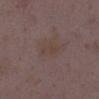biopsy status: catalogued during a skin exam; not biopsied | automated lesion analysis: a mean CIELAB color near L≈40 a*≈15 b*≈20, about 4 CIELAB-L* units darker than the surrounding skin, and a normalized lesion–skin contrast near 4.5; an automated nevus-likeness rating near 0 out of 100 and a lesion-detection confidence of about 100/100 | anatomic site: the left lower leg | patient: female, aged around 35 | image: ~15 mm tile from a whole-body skin photo.